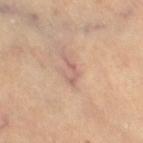  biopsy_status: not biopsied; imaged during a skin examination
  patient:
    sex: female
    age_approx: 70
  lighting: cross-polarized
  image:
    source: total-body photography crop
    field_of_view_mm: 15
  site: right thigh
  lesion_size:
    long_diameter_mm_approx: 3.5